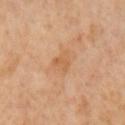Impression:
The lesion was photographed on a routine skin check and not biopsied; there is no pathology result.
Context:
A male patient about 65 years old. Cropped from a whole-body photographic skin survey; the tile spans about 15 mm.